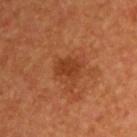The lesion was photographed on a routine skin check and not biopsied; there is no pathology result.